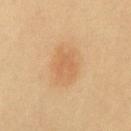Imaged during a routine full-body skin examination; the lesion was not biopsied and no histopathology is available. On the chest. Cropped from a whole-body photographic skin survey; the tile spans about 15 mm. Approximately 4 mm at its widest. Automated tile analysis of the lesion measured an area of roughly 8.5 mm², an eccentricity of roughly 0.7, and two-axis asymmetry of about 0.2. The analysis additionally found a border-irregularity index near 2.5/10, internal color variation of about 2.5 on a 0–10 scale, and radial color variation of about 1. The patient is a female about 25 years old. This is a cross-polarized tile.